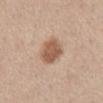workup: catalogued during a skin exam; not biopsied | subject: male, about 55 years old | body site: the mid back | TBP lesion metrics: an average lesion color of about L≈57 a*≈20 b*≈30 (CIELAB), about 13 CIELAB-L* units darker than the surrounding skin, and a lesion-to-skin contrast of about 8.5 (normalized; higher = more distinct); a border-irregularity rating of about 1.5/10, a color-variation rating of about 2.5/10, and peripheral color asymmetry of about 0.5 | imaging modality: ~15 mm crop, total-body skin-cancer survey | illumination: white-light illumination.